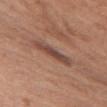Case summary:
• biopsy status: no biopsy performed (imaged during a skin exam)
• patient: female, aged 63–67
• tile lighting: white-light illumination
• acquisition: total-body-photography crop, ~15 mm field of view
• site: the left upper arm
• lesion diameter: about 4.5 mm